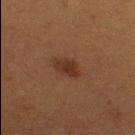notes=no biopsy performed (imaged during a skin exam) | location=the right thigh | TBP lesion metrics=a lesion area of about 6 mm², an eccentricity of roughly 0.75, and a symmetry-axis asymmetry near 0.25; a classifier nevus-likeness of about 80/100 and a lesion-detection confidence of about 100/100 | size=about 3.5 mm | subject=female, aged approximately 40 | image=~15 mm tile from a whole-body skin photo | tile lighting=cross-polarized.